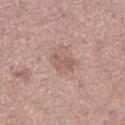Q: Was a biopsy performed?
A: imaged on a skin check; not biopsied
Q: What kind of image is this?
A: ~15 mm tile from a whole-body skin photo
Q: Lesion location?
A: the right lower leg
Q: Automated lesion metrics?
A: a mean CIELAB color near L≈56 a*≈19 b*≈25 and a lesion–skin lightness drop of about 8; a lesion-detection confidence of about 100/100
Q: Patient demographics?
A: female, in their 60s
Q: Lesion size?
A: ≈2.5 mm
Q: How was the tile lit?
A: white-light illumination